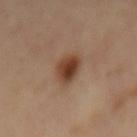Impression: This lesion was catalogued during total-body skin photography and was not selected for biopsy. Image and clinical context: Measured at roughly 3.5 mm in maximum diameter. A 15 mm close-up tile from a total-body photography series done for melanoma screening. From the mid back. Imaged with cross-polarized lighting.